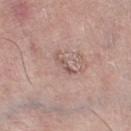Imaged during a routine full-body skin examination; the lesion was not biopsied and no histopathology is available. Imaged with white-light lighting. A region of skin cropped from a whole-body photographic capture, roughly 15 mm wide. The total-body-photography lesion software estimated a detector confidence of about 75 out of 100 that the crop contains a lesion. Measured at roughly 3 mm in maximum diameter. From the leg. The subject is a male about 75 years old.